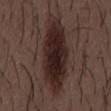Clinical impression: Part of a total-body skin-imaging series; this lesion was reviewed on a skin check and was not flagged for biopsy. Context: Imaged with white-light lighting. From the mid back. The lesion-visualizer software estimated a lesion color around L≈24 a*≈15 b*≈17 in CIELAB, about 11 CIELAB-L* units darker than the surrounding skin, and a normalized lesion–skin contrast near 12. The analysis additionally found a border-irregularity index near 2/10, a color-variation rating of about 5/10, and peripheral color asymmetry of about 1.5. The analysis additionally found a nevus-likeness score of about 100/100 and a detector confidence of about 100 out of 100 that the crop contains a lesion. The patient is a male in their 50s. A 15 mm close-up tile from a total-body photography series done for melanoma screening. Approximately 10 mm at its widest.